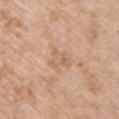This lesion was catalogued during total-body skin photography and was not selected for biopsy. This is a white-light tile. On the left upper arm. Cropped from a total-body skin-imaging series; the visible field is about 15 mm. A female subject, about 70 years old. The lesion's longest dimension is about 3.5 mm.